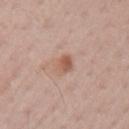notes — total-body-photography surveillance lesion; no biopsy | subject — male, aged approximately 70 | location — the arm | imaging modality — total-body-photography crop, ~15 mm field of view | lesion diameter — ~3 mm (longest diameter) | lighting — white-light illumination | automated lesion analysis — an area of roughly 4.5 mm² and an outline eccentricity of about 0.75 (0 = round, 1 = elongated); a mean CIELAB color near L≈57 a*≈21 b*≈29 and about 9 CIELAB-L* units darker than the surrounding skin; a border-irregularity index near 2/10, a within-lesion color-variation index near 5/10, and radial color variation of about 2.